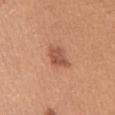notes: no biopsy performed (imaged during a skin exam) | acquisition: total-body-photography crop, ~15 mm field of view | location: the abdomen | image-analysis metrics: an outline eccentricity of about 0.7 (0 = round, 1 = elongated) | patient: male, about 55 years old.